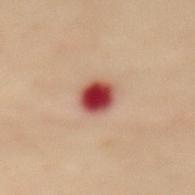On the back.
A 15 mm crop from a total-body photograph taken for skin-cancer surveillance.
The total-body-photography lesion software estimated a lesion area of about 6 mm² and a symmetry-axis asymmetry near 0.05. The software also gave a lesion color around L≈39 a*≈31 b*≈25 in CIELAB, roughly 20 lightness units darker than nearby skin, and a normalized border contrast of about 15. The analysis additionally found a border-irregularity rating of about 0.5/10 and radial color variation of about 1.5. The analysis additionally found a lesion-detection confidence of about 100/100.
The patient is a female aged 58 to 62.